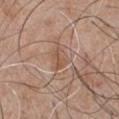Clinical impression: Recorded during total-body skin imaging; not selected for excision or biopsy. Clinical summary: A 15 mm close-up extracted from a 3D total-body photography capture. The patient is a male roughly 50 years of age. The recorded lesion diameter is about 3 mm. The lesion is located on the chest. Automated image analysis of the tile measured a mean CIELAB color near L≈53 a*≈19 b*≈29 and a lesion–skin lightness drop of about 7. It also reported a border-irregularity rating of about 5.5/10, a within-lesion color-variation index near 0/10, and radial color variation of about 0. The analysis additionally found a nevus-likeness score of about 0/100 and lesion-presence confidence of about 70/100. This is a white-light tile.